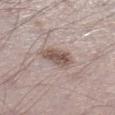Assessment: Recorded during total-body skin imaging; not selected for excision or biopsy. Clinical summary: The lesion is on the left lower leg. A 15 mm close-up extracted from a 3D total-body photography capture. Longest diameter approximately 4 mm. This is a white-light tile. The patient is a male in their 70s.